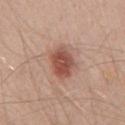A male subject in their 50s. A lesion tile, about 15 mm wide, cut from a 3D total-body photograph. From the front of the torso.